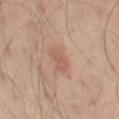Impression:
The lesion was tiled from a total-body skin photograph and was not biopsied.
Image and clinical context:
A male patient aged 48 to 52. Longest diameter approximately 3.5 mm. Automated image analysis of the tile measured a lesion color around L≈56 a*≈21 b*≈27 in CIELAB and roughly 7 lightness units darker than nearby skin. This image is a 15 mm lesion crop taken from a total-body photograph. The lesion is on the leg. Captured under cross-polarized illumination.